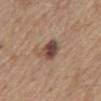Clinical impression:
The lesion was photographed on a routine skin check and not biopsied; there is no pathology result.
Acquisition and patient details:
Automated tile analysis of the lesion measured a mean CIELAB color near L≈45 a*≈18 b*≈25 and roughly 14 lightness units darker than nearby skin. The software also gave a border-irregularity rating of about 2/10. It also reported a classifier nevus-likeness of about 90/100 and lesion-presence confidence of about 100/100. The patient is a male aged 68–72. Measured at roughly 3.5 mm in maximum diameter. Located on the mid back. Cropped from a whole-body photographic skin survey; the tile spans about 15 mm. This is a white-light tile.